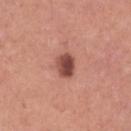  biopsy_status: not biopsied; imaged during a skin examination
  image:
    source: total-body photography crop
    field_of_view_mm: 15
  patient:
    sex: female
    age_approx: 30
  lighting: white-light
  site: right lower leg
  automated_metrics:
    area_mm2_approx: 5.5
    eccentricity: 0.6
    shape_asymmetry: 0.2
    cielab_L: 47
    cielab_a: 26
    cielab_b: 26
    vs_skin_darker_L: 16.0
    vs_skin_contrast_norm: 11.0
    nevus_likeness_0_100: 65